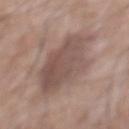follow-up: no biopsy performed (imaged during a skin exam) | lesion diameter: ≈7 mm | imaging modality: ~15 mm crop, total-body skin-cancer survey | patient: male, aged approximately 70 | body site: the abdomen | image-analysis metrics: a footprint of about 27 mm², an outline eccentricity of about 0.6 (0 = round, 1 = elongated), and two-axis asymmetry of about 0.25; a lesion–skin lightness drop of about 10; an automated nevus-likeness rating near 0 out of 100 and a detector confidence of about 100 out of 100 that the crop contains a lesion.